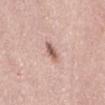| feature | finding |
|---|---|
| biopsy status | no biopsy performed (imaged during a skin exam) |
| size | about 2.5 mm |
| automated metrics | a lesion area of about 3.5 mm² and an outline eccentricity of about 0.8 (0 = round, 1 = elongated); a mean CIELAB color near L≈59 a*≈20 b*≈25 and a normalized border contrast of about 8.5; a border-irregularity rating of about 2/10 |
| subject | female, aged around 35 |
| image source | ~15 mm tile from a whole-body skin photo |
| tile lighting | white-light illumination |
| site | the lower back |